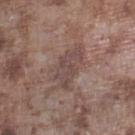<tbp_lesion>
  <biopsy_status>not biopsied; imaged during a skin examination</biopsy_status>
  <lesion_size>
    <long_diameter_mm_approx>5.0</long_diameter_mm_approx>
  </lesion_size>
  <patient>
    <sex>male</sex>
    <age_approx>75</age_approx>
  </patient>
  <site>left lower leg</site>
  <image>
    <source>total-body photography crop</source>
    <field_of_view_mm>15</field_of_view_mm>
  </image>
  <lighting>white-light</lighting>
</tbp_lesion>